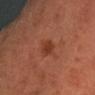| feature | finding |
|---|---|
| follow-up | no biopsy performed (imaged during a skin exam) |
| lighting | cross-polarized |
| imaging modality | 15 mm crop, total-body photography |
| body site | the right forearm |
| subject | male, aged around 60 |